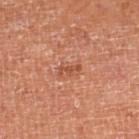Part of a total-body skin-imaging series; this lesion was reviewed on a skin check and was not flagged for biopsy.
The tile uses cross-polarized illumination.
Longest diameter approximately 2.5 mm.
From the leg.
A 15 mm close-up tile from a total-body photography series done for melanoma screening.
Automated image analysis of the tile measured a footprint of about 2.5 mm² and a shape eccentricity near 0.9. It also reported a lesion color around L≈53 a*≈29 b*≈35 in CIELAB, roughly 9 lightness units darker than nearby skin, and a lesion-to-skin contrast of about 6.5 (normalized; higher = more distinct). The analysis additionally found a border-irregularity index near 5.5/10 and a within-lesion color-variation index near 0/10. The software also gave a nevus-likeness score of about 10/100 and a detector confidence of about 100 out of 100 that the crop contains a lesion.
The subject is female.